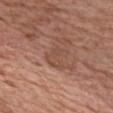Recorded during total-body skin imaging; not selected for excision or biopsy.
Imaged with white-light lighting.
About 3.5 mm across.
The lesion is on the chest.
A region of skin cropped from a whole-body photographic capture, roughly 15 mm wide.
The lesion-visualizer software estimated a footprint of about 5 mm², a shape eccentricity near 0.8, and two-axis asymmetry of about 0.5. The analysis additionally found a mean CIELAB color near L≈48 a*≈20 b*≈28, about 6 CIELAB-L* units darker than the surrounding skin, and a lesion-to-skin contrast of about 4.5 (normalized; higher = more distinct). It also reported border irregularity of about 6.5 on a 0–10 scale, a color-variation rating of about 3/10, and peripheral color asymmetry of about 1. The analysis additionally found a lesion-detection confidence of about 100/100.
The subject is a male approximately 60 years of age.